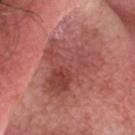notes: catalogued during a skin exam; not biopsied
image-analysis metrics: a lesion–skin lightness drop of about 9 and a lesion-to-skin contrast of about 6.5 (normalized; higher = more distinct); an automated nevus-likeness rating near 0 out of 100 and a lesion-detection confidence of about 100/100
body site: the head or neck
imaging modality: ~15 mm crop, total-body skin-cancer survey
diameter: ~7 mm (longest diameter)
patient: male, aged 23 to 27
tile lighting: white-light